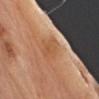Findings:
• patient — male, about 70 years old
• image — 15 mm crop, total-body photography